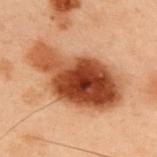– subject · male, in their mid- to late 50s
– image source · ~15 mm tile from a whole-body skin photo
– anatomic site · the upper back